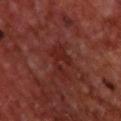workup — imaged on a skin check; not biopsied | lesion size — ≈4 mm | location — the upper back | imaging modality — ~15 mm crop, total-body skin-cancer survey | lighting — cross-polarized | patient — male, aged 68 to 72.